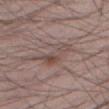Q: Is there a histopathology result?
A: catalogued during a skin exam; not biopsied
Q: What kind of image is this?
A: ~15 mm tile from a whole-body skin photo
Q: How large is the lesion?
A: about 5.5 mm
Q: Who is the patient?
A: male, aged approximately 50
Q: Lesion location?
A: the right thigh
Q: Automated lesion metrics?
A: a footprint of about 9 mm², an outline eccentricity of about 0.9 (0 = round, 1 = elongated), and two-axis asymmetry of about 0.5; a mean CIELAB color near L≈48 a*≈15 b*≈20, roughly 7 lightness units darker than nearby skin, and a lesion-to-skin contrast of about 6 (normalized; higher = more distinct); a border-irregularity index near 7/10, internal color variation of about 6.5 on a 0–10 scale, and radial color variation of about 2; a nevus-likeness score of about 0/100 and a lesion-detection confidence of about 90/100